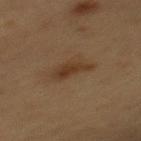Impression:
No biopsy was performed on this lesion — it was imaged during a full skin examination and was not determined to be concerning.
Background:
The total-body-photography lesion software estimated an area of roughly 5.5 mm², an outline eccentricity of about 0.9 (0 = round, 1 = elongated), and a shape-asymmetry score of about 0.4 (0 = symmetric). The analysis additionally found a lesion color around L≈30 a*≈14 b*≈26 in CIELAB, a lesion–skin lightness drop of about 7, and a normalized border contrast of about 7.5. The analysis additionally found an automated nevus-likeness rating near 50 out of 100. The lesion's longest dimension is about 4 mm. A 15 mm crop from a total-body photograph taken for skin-cancer surveillance. A female patient about 40 years old. The lesion is located on the mid back. This is a cross-polarized tile.